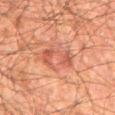Clinical impression: Part of a total-body skin-imaging series; this lesion was reviewed on a skin check and was not flagged for biopsy. Background: Imaged with cross-polarized lighting. About 4 mm across. On the mid back. A roughly 15 mm field-of-view crop from a total-body skin photograph. A male patient, aged 58 to 62.